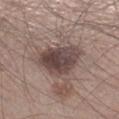image=total-body-photography crop, ~15 mm field of view; patient=male, in their mid-40s; location=the right lower leg; lighting=white-light illumination.